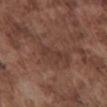• follow-up — no biopsy performed (imaged during a skin exam)
• image — total-body-photography crop, ~15 mm field of view
• subject — male, approximately 75 years of age
• lesion diameter — ≈3.5 mm
• body site — the chest
• illumination — white-light illumination